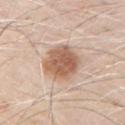- biopsy status — catalogued during a skin exam; not biopsied
- illumination — white-light illumination
- anatomic site — the left upper arm
- image — ~15 mm tile from a whole-body skin photo
- lesion diameter — about 4.5 mm
- patient — male, in their 70s